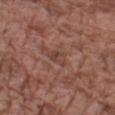Imaged during a routine full-body skin examination; the lesion was not biopsied and no histopathology is available.
About 3 mm across.
The lesion is located on the arm.
Cropped from a total-body skin-imaging series; the visible field is about 15 mm.
Automated image analysis of the tile measured a lesion area of about 4 mm² and a symmetry-axis asymmetry near 0.4. And it measured border irregularity of about 3.5 on a 0–10 scale and peripheral color asymmetry of about 1.5. The analysis additionally found a classifier nevus-likeness of about 0/100 and a lesion-detection confidence of about 95/100.
The subject is a male about 75 years old.